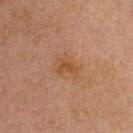workup: total-body-photography surveillance lesion; no biopsy
acquisition: ~15 mm tile from a whole-body skin photo
TBP lesion metrics: an area of roughly 4.5 mm², an outline eccentricity of about 0.7 (0 = round, 1 = elongated), and a symmetry-axis asymmetry near 0.5; an average lesion color of about L≈43 a*≈20 b*≈31 (CIELAB) and a lesion–skin lightness drop of about 6; a border-irregularity rating of about 5/10, a color-variation rating of about 1.5/10, and radial color variation of about 0.5; an automated nevus-likeness rating near 5 out of 100 and lesion-presence confidence of about 100/100
diameter: ≈3 mm
patient: female, in their mid- to late 40s
body site: the head or neck